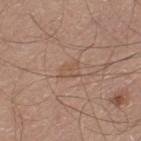Clinical impression:
No biopsy was performed on this lesion — it was imaged during a full skin examination and was not determined to be concerning.
Clinical summary:
On the left thigh. The recorded lesion diameter is about 2.5 mm. This is a white-light tile. This image is a 15 mm lesion crop taken from a total-body photograph. A male subject, about 55 years old.